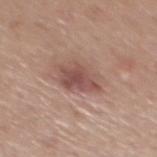biopsy status = imaged on a skin check; not biopsied | location = the mid back | image source = total-body-photography crop, ~15 mm field of view | tile lighting = white-light illumination | image-analysis metrics = a lesion color around L≈50 a*≈21 b*≈23 in CIELAB; an automated nevus-likeness rating near 65 out of 100 and lesion-presence confidence of about 100/100 | patient = male, about 50 years old.